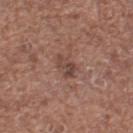The lesion was photographed on a routine skin check and not biopsied; there is no pathology result. About 3 mm across. A region of skin cropped from a whole-body photographic capture, roughly 15 mm wide. A female subject, roughly 50 years of age. Imaged with white-light lighting. The lesion is located on the left thigh.